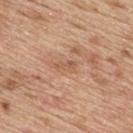Assessment:
Imaged during a routine full-body skin examination; the lesion was not biopsied and no histopathology is available.
Background:
The lesion's longest dimension is about 2.5 mm. Cropped from a total-body skin-imaging series; the visible field is about 15 mm. Located on the upper back. The lesion-visualizer software estimated a color-variation rating of about 0.5/10. The patient is a male about 70 years old.